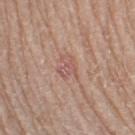Impression: The lesion was tiled from a total-body skin photograph and was not biopsied. Clinical summary: The lesion's longest dimension is about 3 mm. A lesion tile, about 15 mm wide, cut from a 3D total-body photograph. A female patient about 70 years old. Captured under white-light illumination. From the right thigh.